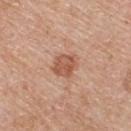Imaged during a routine full-body skin examination; the lesion was not biopsied and no histopathology is available. A 15 mm close-up extracted from a 3D total-body photography capture. An algorithmic analysis of the crop reported a nevus-likeness score of about 35/100 and a detector confidence of about 100 out of 100 that the crop contains a lesion. This is a white-light tile. Longest diameter approximately 3 mm. From the back. The subject is a male aged 58–62.